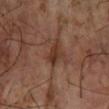Captured during whole-body skin photography for melanoma surveillance; the lesion was not biopsied.
A close-up tile cropped from a whole-body skin photograph, about 15 mm across.
The patient is a male aged approximately 65.
On the arm.
Measured at roughly 3 mm in maximum diameter.